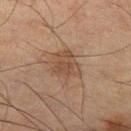subject — male, in their mid-60s | anatomic site — the left thigh | image source — ~15 mm crop, total-body skin-cancer survey.